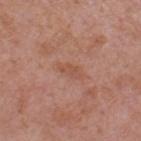This lesion was catalogued during total-body skin photography and was not selected for biopsy.
The lesion is on the right thigh.
Cropped from a whole-body photographic skin survey; the tile spans about 15 mm.
A female subject, aged 38–42.